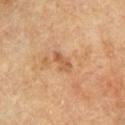| feature | finding |
|---|---|
| lighting | cross-polarized |
| automated lesion analysis | an area of roughly 3.5 mm², an outline eccentricity of about 0.85 (0 = round, 1 = elongated), and a shape-asymmetry score of about 0.3 (0 = symmetric); a normalized lesion–skin contrast near 6; an automated nevus-likeness rating near 0 out of 100 and a detector confidence of about 100 out of 100 that the crop contains a lesion |
| location | the chest |
| lesion diameter | ≈3 mm |
| subject | male, roughly 65 years of age |
| acquisition | 15 mm crop, total-body photography |